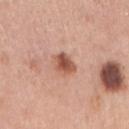follow-up: no biopsy performed (imaged during a skin exam); automated lesion analysis: a classifier nevus-likeness of about 95/100 and a lesion-detection confidence of about 100/100; diameter: ~2.5 mm (longest diameter); patient: female, aged 58 to 62; site: the right upper arm; acquisition: ~15 mm crop, total-body skin-cancer survey; lighting: white-light illumination.